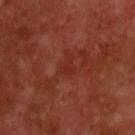workup: catalogued during a skin exam; not biopsied.